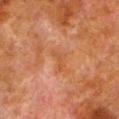workup = total-body-photography surveillance lesion; no biopsy
body site = the left lower leg
subject = male, aged approximately 80
illumination = cross-polarized illumination
size = ~2.5 mm (longest diameter)
imaging modality = 15 mm crop, total-body photography